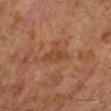Captured during whole-body skin photography for melanoma surveillance; the lesion was not biopsied.
A 15 mm close-up tile from a total-body photography series done for melanoma screening.
A male subject aged around 60.
Approximately 4 mm at its widest.
The lesion is located on the left lower leg.
Imaged with cross-polarized lighting.